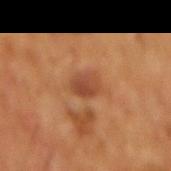Part of a total-body skin-imaging series; this lesion was reviewed on a skin check and was not flagged for biopsy. The lesion's longest dimension is about 3 mm. On the abdomen. A 15 mm crop from a total-body photograph taken for skin-cancer surveillance. The patient is a male aged around 60. This is a cross-polarized tile.